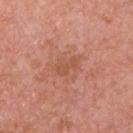biopsy status = total-body-photography surveillance lesion; no biopsy | acquisition = ~15 mm crop, total-body skin-cancer survey | patient = male, roughly 70 years of age | illumination = white-light illumination | site = the chest.